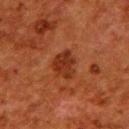The lesion was photographed on a routine skin check and not biopsied; there is no pathology result.
Cropped from a whole-body photographic skin survey; the tile spans about 15 mm.
The lesion is located on the upper back.
A female patient, aged 48 to 52.